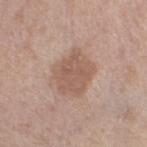- biopsy status: imaged on a skin check; not biopsied
- acquisition: 15 mm crop, total-body photography
- patient: male, aged approximately 80
- anatomic site: the left thigh